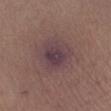<tbp_lesion>
  <biopsy_status>not biopsied; imaged during a skin examination</biopsy_status>
  <patient>
    <sex>female</sex>
    <age_approx>45</age_approx>
  </patient>
  <lesion_size>
    <long_diameter_mm_approx>5.0</long_diameter_mm_approx>
  </lesion_size>
  <image>
    <source>total-body photography crop</source>
    <field_of_view_mm>15</field_of_view_mm>
  </image>
  <site>left lower leg</site>
  <lighting>white-light</lighting>
</tbp_lesion>